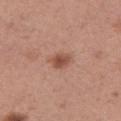Q: Is there a histopathology result?
A: total-body-photography surveillance lesion; no biopsy
Q: Who is the patient?
A: female, approximately 30 years of age
Q: What kind of image is this?
A: ~15 mm crop, total-body skin-cancer survey
Q: How was the tile lit?
A: white-light illumination
Q: How large is the lesion?
A: ≈2.5 mm
Q: Automated lesion metrics?
A: a border-irregularity rating of about 1.5/10, internal color variation of about 2.5 on a 0–10 scale, and peripheral color asymmetry of about 0.5; an automated nevus-likeness rating near 90 out of 100 and a lesion-detection confidence of about 100/100
Q: What is the anatomic site?
A: the right thigh